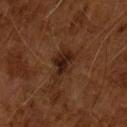workup = total-body-photography surveillance lesion; no biopsy | image source = ~15 mm tile from a whole-body skin photo | patient = male, approximately 65 years of age.